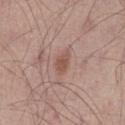Q: Was a biopsy performed?
A: imaged on a skin check; not biopsied
Q: What is the anatomic site?
A: the right thigh
Q: What is the imaging modality?
A: ~15 mm tile from a whole-body skin photo
Q: Lesion size?
A: ≈2.5 mm
Q: Who is the patient?
A: male, in their mid-50s
Q: What lighting was used for the tile?
A: white-light